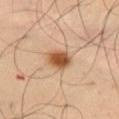follow-up: imaged on a skin check; not biopsied
acquisition: total-body-photography crop, ~15 mm field of view
location: the abdomen
lesion diameter: ~3.5 mm (longest diameter)
subject: male, aged 53–57
lighting: cross-polarized